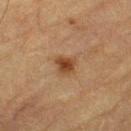lesion size — ≈2.5 mm | illumination — cross-polarized | patient — male, about 85 years old or older | location — the left thigh | imaging modality — ~15 mm tile from a whole-body skin photo | automated metrics — an area of roughly 4.5 mm², an outline eccentricity of about 0.45 (0 = round, 1 = elongated), and a symmetry-axis asymmetry near 0.3; a mean CIELAB color near L≈39 a*≈19 b*≈32 and a lesion-to-skin contrast of about 9 (normalized; higher = more distinct); a border-irregularity rating of about 3/10, internal color variation of about 3 on a 0–10 scale, and peripheral color asymmetry of about 1; lesion-presence confidence of about 100/100.